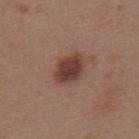Part of a total-body skin-imaging series; this lesion was reviewed on a skin check and was not flagged for biopsy.
Located on the upper back.
A female patient, about 45 years old.
This is a white-light tile.
Measured at roughly 4 mm in maximum diameter.
A 15 mm close-up tile from a total-body photography series done for melanoma screening.
The lesion-visualizer software estimated a footprint of about 9 mm², an outline eccentricity of about 0.7 (0 = round, 1 = elongated), and two-axis asymmetry of about 0.15. The analysis additionally found a color-variation rating of about 3.5/10 and radial color variation of about 1.